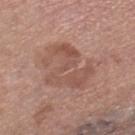Clinical impression:
This lesion was catalogued during total-body skin photography and was not selected for biopsy.
Clinical summary:
From the right lower leg. The subject is a female aged 58–62. A 15 mm crop from a total-body photograph taken for skin-cancer surveillance.